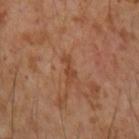follow-up = no biopsy performed (imaged during a skin exam)
lesion diameter = about 2.5 mm
body site = the right forearm
imaging modality = ~15 mm crop, total-body skin-cancer survey
patient = male, aged 53 to 57